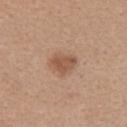Imaged during a routine full-body skin examination; the lesion was not biopsied and no histopathology is available. The lesion is on the chest. A female subject, aged approximately 40. A 15 mm close-up extracted from a 3D total-body photography capture.